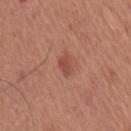Case summary:
– notes · no biopsy performed (imaged during a skin exam)
– anatomic site · the chest
– patient · male, aged 63–67
– image source · total-body-photography crop, ~15 mm field of view